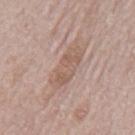Clinical impression: Captured during whole-body skin photography for melanoma surveillance; the lesion was not biopsied. Background: A roughly 15 mm field-of-view crop from a total-body skin photograph. The lesion is on the mid back. Automated tile analysis of the lesion measured a lesion color around L≈57 a*≈17 b*≈26 in CIELAB, roughly 8 lightness units darker than nearby skin, and a lesion-to-skin contrast of about 6 (normalized; higher = more distinct). The software also gave a within-lesion color-variation index near 3/10. The subject is a male aged approximately 70. The recorded lesion diameter is about 6 mm.